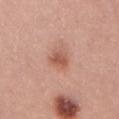No biopsy was performed on this lesion — it was imaged during a full skin examination and was not determined to be concerning. The total-body-photography lesion software estimated a border-irregularity rating of about 2/10, a color-variation rating of about 4.5/10, and a peripheral color-asymmetry measure near 1.5. A close-up tile cropped from a whole-body skin photograph, about 15 mm across. A female subject, in their mid-20s. From the back. Measured at roughly 3 mm in maximum diameter.